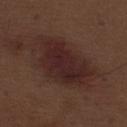The lesion was photographed on a routine skin check and not biopsied; there is no pathology result. This image is a 15 mm lesion crop taken from a total-body photograph. About 8.5 mm across. Automated tile analysis of the lesion measured a shape eccentricity near 0.8. And it measured a lesion color around L≈25 a*≈19 b*≈19 in CIELAB, about 8 CIELAB-L* units darker than the surrounding skin, and a lesion-to-skin contrast of about 9 (normalized; higher = more distinct). The analysis additionally found a border-irregularity rating of about 3.5/10 and internal color variation of about 3.5 on a 0–10 scale. The software also gave a nevus-likeness score of about 95/100 and a detector confidence of about 100 out of 100 that the crop contains a lesion. A male patient aged approximately 70. The lesion is on the left thigh.